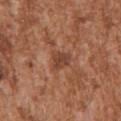Impression:
Part of a total-body skin-imaging series; this lesion was reviewed on a skin check and was not flagged for biopsy.
Acquisition and patient details:
The tile uses white-light illumination. The lesion's longest dimension is about 3 mm. The lesion is located on the left upper arm. A region of skin cropped from a whole-body photographic capture, roughly 15 mm wide. A male subject, aged approximately 45. An algorithmic analysis of the crop reported a lesion area of about 4.5 mm², an outline eccentricity of about 0.7 (0 = round, 1 = elongated), and a shape-asymmetry score of about 0.3 (0 = symmetric). It also reported an automated nevus-likeness rating near 0 out of 100 and lesion-presence confidence of about 100/100.